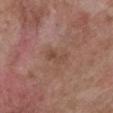Clinical impression: The lesion was photographed on a routine skin check and not biopsied; there is no pathology result. Acquisition and patient details: A male subject, aged 48–52. From the chest. The recorded lesion diameter is about 3 mm. The tile uses white-light illumination. A region of skin cropped from a whole-body photographic capture, roughly 15 mm wide.